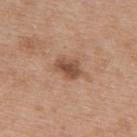biopsy status: catalogued during a skin exam; not biopsied | acquisition: ~15 mm crop, total-body skin-cancer survey | anatomic site: the upper back | subject: female, aged around 40.